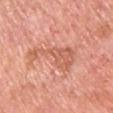Part of a total-body skin-imaging series; this lesion was reviewed on a skin check and was not flagged for biopsy.
This is a white-light tile.
Cropped from a whole-body photographic skin survey; the tile spans about 15 mm.
The recorded lesion diameter is about 7 mm.
The lesion is on the front of the torso.
A male patient, roughly 60 years of age.